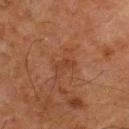| key | value |
|---|---|
| notes | total-body-photography surveillance lesion; no biopsy |
| automated lesion analysis | internal color variation of about 1 on a 0–10 scale and peripheral color asymmetry of about 0.5 |
| patient | male, aged 78 to 82 |
| tile lighting | cross-polarized illumination |
| lesion size | ~2.5 mm (longest diameter) |
| image | total-body-photography crop, ~15 mm field of view |
| body site | the left thigh |